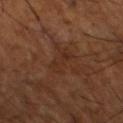Clinical impression:
Recorded during total-body skin imaging; not selected for excision or biopsy.
Context:
The lesion is on the left lower leg. The subject is a male approximately 65 years of age. Approximately 3 mm at its widest. An algorithmic analysis of the crop reported an automated nevus-likeness rating near 0 out of 100 and a detector confidence of about 90 out of 100 that the crop contains a lesion. A region of skin cropped from a whole-body photographic capture, roughly 15 mm wide.